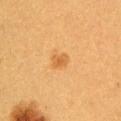No biopsy was performed on this lesion — it was imaged during a full skin examination and was not determined to be concerning.
From the left upper arm.
A region of skin cropped from a whole-body photographic capture, roughly 15 mm wide.
A female subject aged approximately 30.
About 2 mm across.